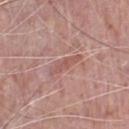lighting: white-light
image-analysis metrics: a lesion area of about 4.5 mm², an outline eccentricity of about 0.95 (0 = round, 1 = elongated), and a symmetry-axis asymmetry near 0.3; a border-irregularity rating of about 4.5/10 and a color-variation rating of about 2.5/10; a classifier nevus-likeness of about 0/100 and a lesion-detection confidence of about 70/100
subject: male, aged around 70
image source: total-body-photography crop, ~15 mm field of view
location: the chest
lesion diameter: about 4.5 mm